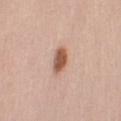Clinical summary:
A 15 mm close-up extracted from a 3D total-body photography capture. Automated image analysis of the tile measured a color-variation rating of about 3/10 and radial color variation of about 1. A female patient, about 50 years old. On the left upper arm.